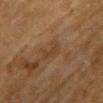Assessment:
Part of a total-body skin-imaging series; this lesion was reviewed on a skin check and was not flagged for biopsy.
Clinical summary:
Located on the upper back. A lesion tile, about 15 mm wide, cut from a 3D total-body photograph. The subject is a female roughly 55 years of age. Approximately 3 mm at its widest. Automated image analysis of the tile measured a footprint of about 4 mm², an outline eccentricity of about 0.85 (0 = round, 1 = elongated), and two-axis asymmetry of about 0.4. The software also gave a nevus-likeness score of about 0/100 and a detector confidence of about 90 out of 100 that the crop contains a lesion. The tile uses cross-polarized illumination.